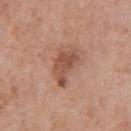notes=catalogued during a skin exam; not biopsied | diameter=≈4.5 mm | image=total-body-photography crop, ~15 mm field of view | patient=male, approximately 70 years of age | body site=the chest | lighting=white-light illumination.